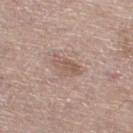The lesion was tiled from a total-body skin photograph and was not biopsied. From the left lower leg. The subject is a male aged 68 to 72. Cropped from a total-body skin-imaging series; the visible field is about 15 mm. Measured at roughly 3.5 mm in maximum diameter. The tile uses white-light illumination. Automated image analysis of the tile measured a lesion area of about 5.5 mm², an outline eccentricity of about 0.85 (0 = round, 1 = elongated), and two-axis asymmetry of about 0.25. The software also gave border irregularity of about 3 on a 0–10 scale, a within-lesion color-variation index near 2.5/10, and a peripheral color-asymmetry measure near 1. It also reported a detector confidence of about 100 out of 100 that the crop contains a lesion.